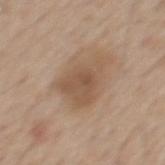Captured during whole-body skin photography for melanoma surveillance; the lesion was not biopsied.
A 15 mm close-up extracted from a 3D total-body photography capture.
The total-body-photography lesion software estimated a footprint of about 14 mm², an outline eccentricity of about 0.7 (0 = round, 1 = elongated), and a symmetry-axis asymmetry near 0.45. It also reported a within-lesion color-variation index near 3/10 and peripheral color asymmetry of about 1. The software also gave a nevus-likeness score of about 5/100 and a detector confidence of about 100 out of 100 that the crop contains a lesion.
The lesion is on the mid back.
Captured under white-light illumination.
A male patient, aged around 55.
About 6 mm across.